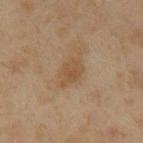Assessment:
Part of a total-body skin-imaging series; this lesion was reviewed on a skin check and was not flagged for biopsy.
Clinical summary:
A male subject about 60 years old. This is a cross-polarized tile. The lesion is located on the abdomen. Measured at roughly 3.5 mm in maximum diameter. This image is a 15 mm lesion crop taken from a total-body photograph.